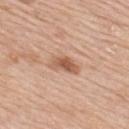Q: Was this lesion biopsied?
A: no biopsy performed (imaged during a skin exam)
Q: How large is the lesion?
A: ~4 mm (longest diameter)
Q: Illumination type?
A: white-light illumination
Q: Lesion location?
A: the upper back
Q: What is the imaging modality?
A: total-body-photography crop, ~15 mm field of view
Q: Who is the patient?
A: male, aged 58 to 62